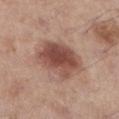notes = total-body-photography surveillance lesion; no biopsy
image = total-body-photography crop, ~15 mm field of view
subject = male, about 55 years old
illumination = white-light illumination
body site = the left lower leg